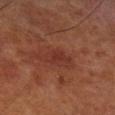Captured during whole-body skin photography for melanoma surveillance; the lesion was not biopsied. The subject is a male aged 68–72. The lesion is on the leg. A 15 mm close-up tile from a total-body photography series done for melanoma screening. Approximately 3 mm at its widest.